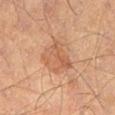Captured during whole-body skin photography for melanoma surveillance; the lesion was not biopsied. About 4 mm across. The lesion-visualizer software estimated an area of roughly 12 mm², an eccentricity of roughly 0.3, and a symmetry-axis asymmetry near 0.3. And it measured a lesion color around L≈55 a*≈21 b*≈33 in CIELAB and about 7 CIELAB-L* units darker than the surrounding skin. The software also gave a border-irregularity rating of about 3/10, a color-variation rating of about 4/10, and a peripheral color-asymmetry measure near 1.5. The analysis additionally found an automated nevus-likeness rating near 5 out of 100 and a lesion-detection confidence of about 100/100. From the left thigh. A male patient aged 53–57. Cropped from a whole-body photographic skin survey; the tile spans about 15 mm. Captured under cross-polarized illumination.